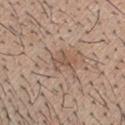Q: Was this lesion biopsied?
A: imaged on a skin check; not biopsied
Q: What kind of image is this?
A: 15 mm crop, total-body photography
Q: Illumination type?
A: white-light illumination
Q: What did automated image analysis measure?
A: an area of roughly 3.5 mm² and a shape-asymmetry score of about 0.4 (0 = symmetric); border irregularity of about 4.5 on a 0–10 scale, internal color variation of about 0.5 on a 0–10 scale, and radial color variation of about 0
Q: What is the anatomic site?
A: the head or neck
Q: Who is the patient?
A: male, aged 28 to 32